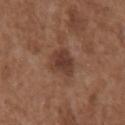workup: catalogued during a skin exam; not biopsied | patient: male, in their mid- to late 70s | body site: the chest | acquisition: ~15 mm crop, total-body skin-cancer survey.